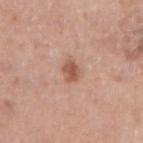Q: Was this lesion biopsied?
A: imaged on a skin check; not biopsied
Q: How was this image acquired?
A: total-body-photography crop, ~15 mm field of view
Q: What is the anatomic site?
A: the right upper arm
Q: Who is the patient?
A: male, aged around 75
Q: What did automated image analysis measure?
A: a border-irregularity index near 2/10, a within-lesion color-variation index near 2/10, and peripheral color asymmetry of about 0.5; an automated nevus-likeness rating near 85 out of 100 and lesion-presence confidence of about 100/100
Q: Lesion size?
A: ≈2.5 mm
Q: What lighting was used for the tile?
A: white-light